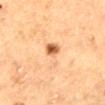Q: Is there a histopathology result?
A: imaged on a skin check; not biopsied
Q: Who is the patient?
A: female, aged around 60
Q: Automated lesion metrics?
A: a lesion area of about 6.5 mm² and an outline eccentricity of about 0.7 (0 = round, 1 = elongated)
Q: Illumination type?
A: cross-polarized
Q: Lesion location?
A: the mid back
Q: How was this image acquired?
A: ~15 mm tile from a whole-body skin photo